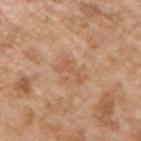Case summary:
- workup: catalogued during a skin exam; not biopsied
- diameter: about 3.5 mm
- patient: male, approximately 65 years of age
- acquisition: ~15 mm tile from a whole-body skin photo
- body site: the arm
- illumination: white-light